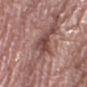Recorded during total-body skin imaging; not selected for excision or biopsy.
A 15 mm crop from a total-body photograph taken for skin-cancer surveillance.
Located on the right lower leg.
The total-body-photography lesion software estimated an average lesion color of about L≈45 a*≈22 b*≈21 (CIELAB), a lesion–skin lightness drop of about 12, and a normalized border contrast of about 9. And it measured a classifier nevus-likeness of about 0/100 and a lesion-detection confidence of about 70/100.
A female patient, roughly 65 years of age.
The lesion's longest dimension is about 4.5 mm.